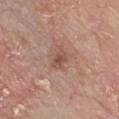Clinical impression: The lesion was photographed on a routine skin check and not biopsied; there is no pathology result. Acquisition and patient details: On the front of the torso. The patient is a male in their 80s. Measured at roughly 2.5 mm in maximum diameter. A 15 mm close-up extracted from a 3D total-body photography capture.